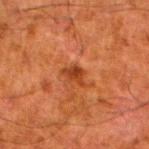Part of a total-body skin-imaging series; this lesion was reviewed on a skin check and was not flagged for biopsy. A male patient, approximately 80 years of age. A 15 mm close-up extracted from a 3D total-body photography capture. The lesion is located on the leg.